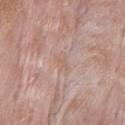- workup — imaged on a skin check; not biopsied
- patient — male, approximately 55 years of age
- tile lighting — white-light illumination
- anatomic site — the mid back
- lesion diameter — about 2.5 mm
- imaging modality — 15 mm crop, total-body photography